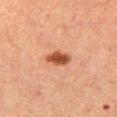Clinical impression: Captured during whole-body skin photography for melanoma surveillance; the lesion was not biopsied. Image and clinical context: An algorithmic analysis of the crop reported a footprint of about 5 mm², a shape eccentricity near 0.85, and a symmetry-axis asymmetry near 0.15. The software also gave roughly 14 lightness units darker than nearby skin and a normalized lesion–skin contrast near 11. And it measured a border-irregularity rating of about 1.5/10, a within-lesion color-variation index near 2/10, and peripheral color asymmetry of about 0.5. And it measured lesion-presence confidence of about 100/100. The lesion's longest dimension is about 3.5 mm. Located on the left thigh. The tile uses cross-polarized illumination. A region of skin cropped from a whole-body photographic capture, roughly 15 mm wide. A female patient aged approximately 40.